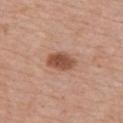Captured during whole-body skin photography for melanoma surveillance; the lesion was not biopsied. From the chest. A lesion tile, about 15 mm wide, cut from a 3D total-body photograph. The patient is a female about 75 years old.